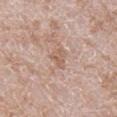Clinical impression:
Captured during whole-body skin photography for melanoma surveillance; the lesion was not biopsied.
Acquisition and patient details:
About 3 mm across. A male patient approximately 60 years of age. An algorithmic analysis of the crop reported a lesion color around L≈59 a*≈19 b*≈28 in CIELAB, about 7 CIELAB-L* units darker than the surrounding skin, and a normalized lesion–skin contrast near 5.5. The software also gave border irregularity of about 4 on a 0–10 scale. From the leg. Captured under white-light illumination. A roughly 15 mm field-of-view crop from a total-body skin photograph.